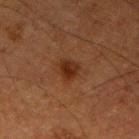Clinical impression: This lesion was catalogued during total-body skin photography and was not selected for biopsy. Context: A 15 mm crop from a total-body photograph taken for skin-cancer surveillance. Imaged with cross-polarized lighting. The total-body-photography lesion software estimated an outline eccentricity of about 0.4 (0 = round, 1 = elongated) and two-axis asymmetry of about 0.3. The software also gave roughly 7 lightness units darker than nearby skin. And it measured a border-irregularity rating of about 2.5/10 and radial color variation of about 1. And it measured an automated nevus-likeness rating near 90 out of 100 and lesion-presence confidence of about 100/100. A male patient, roughly 75 years of age. Located on the right upper arm. Measured at roughly 2.5 mm in maximum diameter.